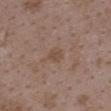follow-up = total-body-photography surveillance lesion; no biopsy